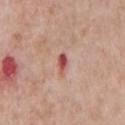Q: What did automated image analysis measure?
A: a border-irregularity rating of about 2/10, a color-variation rating of about 2/10, and radial color variation of about 0.5
Q: Who is the patient?
A: male, aged 58 to 62
Q: How was the tile lit?
A: white-light illumination
Q: Lesion location?
A: the chest
Q: How was this image acquired?
A: ~15 mm crop, total-body skin-cancer survey